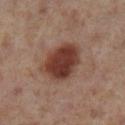follow-up = imaged on a skin check; not biopsied
image source = total-body-photography crop, ~15 mm field of view
automated metrics = an area of roughly 16 mm² and two-axis asymmetry of about 0.2; internal color variation of about 4 on a 0–10 scale and radial color variation of about 1; lesion-presence confidence of about 100/100
location = the leg
subject = female, in their 40s
tile lighting = cross-polarized illumination